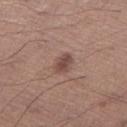Captured during whole-body skin photography for melanoma surveillance; the lesion was not biopsied. A region of skin cropped from a whole-body photographic capture, roughly 15 mm wide. The recorded lesion diameter is about 2.5 mm. The tile uses white-light illumination. The subject is a male aged 63 to 67. From the left thigh. The total-body-photography lesion software estimated two-axis asymmetry of about 0.25.